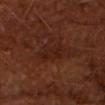Q: Was this lesion biopsied?
A: imaged on a skin check; not biopsied
Q: What is the lesion's diameter?
A: ≈3.5 mm
Q: What is the imaging modality?
A: 15 mm crop, total-body photography
Q: Patient demographics?
A: male, aged around 65
Q: Illumination type?
A: cross-polarized illumination